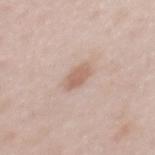Recorded during total-body skin imaging; not selected for excision or biopsy. The lesion is on the mid back. A lesion tile, about 15 mm wide, cut from a 3D total-body photograph. This is a white-light tile. A female patient roughly 40 years of age.